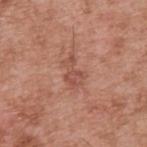Part of a total-body skin-imaging series; this lesion was reviewed on a skin check and was not flagged for biopsy.